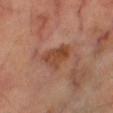Q: Is there a histopathology result?
A: total-body-photography surveillance lesion; no biopsy
Q: What kind of image is this?
A: ~15 mm tile from a whole-body skin photo
Q: What is the anatomic site?
A: the right thigh
Q: What are the patient's age and sex?
A: male, aged around 70